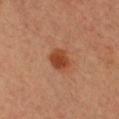This lesion was catalogued during total-body skin photography and was not selected for biopsy. Captured under cross-polarized illumination. This image is a 15 mm lesion crop taken from a total-body photograph. A female patient about 40 years old. Approximately 3 mm at its widest. The lesion is located on the chest.